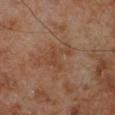Impression:
Recorded during total-body skin imaging; not selected for excision or biopsy.
Context:
The recorded lesion diameter is about 4 mm. This is a cross-polarized tile. The lesion is located on the left lower leg. A 15 mm crop from a total-body photograph taken for skin-cancer surveillance. A male patient, aged 68–72. Automated tile analysis of the lesion measured a footprint of about 8 mm², an outline eccentricity of about 0.7 (0 = round, 1 = elongated), and two-axis asymmetry of about 0.65. The software also gave border irregularity of about 8 on a 0–10 scale.